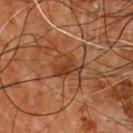Notes:
- location — the chest
- illumination — cross-polarized
- acquisition — ~15 mm tile from a whole-body skin photo
- lesion size — ≈4 mm
- subject — male, aged approximately 55